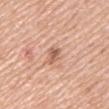Findings:
- notes — imaged on a skin check; not biopsied
- lighting — white-light
- acquisition — ~15 mm crop, total-body skin-cancer survey
- subject — male, aged 53 to 57
- site — the right upper arm
- diameter — ≈2.5 mm